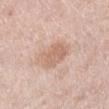| key | value |
|---|---|
| workup | imaged on a skin check; not biopsied |
| location | the leg |
| tile lighting | white-light |
| subject | female, approximately 40 years of age |
| acquisition | total-body-photography crop, ~15 mm field of view |